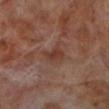Notes:
- automated metrics — an area of roughly 4.5 mm², an eccentricity of roughly 0.7, and two-axis asymmetry of about 0.25; an automated nevus-likeness rating near 0 out of 100 and a lesion-detection confidence of about 100/100
- site — the left lower leg
- image source — 15 mm crop, total-body photography
- size — ≈2.5 mm
- illumination — cross-polarized
- subject — male, in their 70s